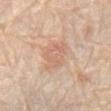The lesion was photographed on a routine skin check and not biopsied; there is no pathology result. A male patient approximately 80 years of age. An algorithmic analysis of the crop reported an average lesion color of about L≈66 a*≈20 b*≈30 (CIELAB), roughly 7 lightness units darker than nearby skin, and a normalized border contrast of about 4.5. It also reported a border-irregularity rating of about 3/10, a color-variation rating of about 3/10, and a peripheral color-asymmetry measure near 1. The software also gave a classifier nevus-likeness of about 5/100 and a lesion-detection confidence of about 100/100. Approximately 4 mm at its widest. The lesion is located on the abdomen. A 15 mm crop from a total-body photograph taken for skin-cancer surveillance. This is a white-light tile.